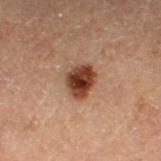Case summary:
- biopsy status — catalogued during a skin exam; not biopsied
- image-analysis metrics — a lesion area of about 7.5 mm², an eccentricity of roughly 0.65, and a symmetry-axis asymmetry near 0.25; a lesion color around L≈29 a*≈19 b*≈24 in CIELAB and a lesion-to-skin contrast of about 13.5 (normalized; higher = more distinct); a border-irregularity rating of about 2.5/10 and a peripheral color-asymmetry measure near 2
- imaging modality — ~15 mm tile from a whole-body skin photo
- patient — male, roughly 70 years of age
- illumination — cross-polarized
- body site — the left lower leg
- lesion diameter — ≈3.5 mm